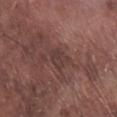Q: Lesion location?
A: the leg
Q: What are the patient's age and sex?
A: male, approximately 75 years of age
Q: How was this image acquired?
A: 15 mm crop, total-body photography